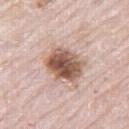Clinical impression: Recorded during total-body skin imaging; not selected for excision or biopsy. Clinical summary: The patient is a male roughly 80 years of age. Captured under white-light illumination. About 4.5 mm across. From the leg. A close-up tile cropped from a whole-body skin photograph, about 15 mm across.